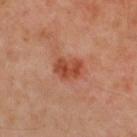Imaged during a routine full-body skin examination; the lesion was not biopsied and no histopathology is available. Imaged with cross-polarized lighting. The recorded lesion diameter is about 3 mm. Located on the upper back. A 15 mm close-up extracted from a 3D total-body photography capture. The patient is a male roughly 50 years of age.